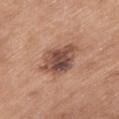site: chest
lighting: white-light
image:
  source: total-body photography crop
  field_of_view_mm: 15
patient:
  sex: female
  age_approx: 65
automated_metrics:
  cielab_L: 48
  cielab_a: 21
  cielab_b: 26
  vs_skin_darker_L: 14.0
  vs_skin_contrast_norm: 10.5
  nevus_likeness_0_100: 15
  lesion_detection_confidence_0_100: 100